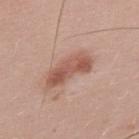Imaged during a routine full-body skin examination; the lesion was not biopsied and no histopathology is available. About 5.5 mm across. A male patient, roughly 40 years of age. Located on the upper back. Captured under white-light illumination. A roughly 15 mm field-of-view crop from a total-body skin photograph.